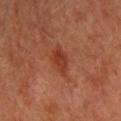follow-up: imaged on a skin check; not biopsied | acquisition: total-body-photography crop, ~15 mm field of view | subject: male, aged 63–67 | anatomic site: the right upper arm.